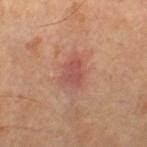The lesion was tiled from a total-body skin photograph and was not biopsied. A region of skin cropped from a whole-body photographic capture, roughly 15 mm wide. A male subject, aged 63 to 67. This is a cross-polarized tile. The lesion's longest dimension is about 3 mm. Located on the right thigh.